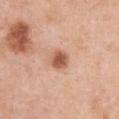Case summary:
* workup · imaged on a skin check; not biopsied
* automated lesion analysis · a normalized border contrast of about 9; a border-irregularity index near 1.5/10 and radial color variation of about 0.5
* subject · female, aged approximately 40
* acquisition · total-body-photography crop, ~15 mm field of view
* illumination · white-light
* size · ≈2.5 mm
* location · the chest